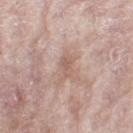Clinical impression:
The lesion was photographed on a routine skin check and not biopsied; there is no pathology result.
Image and clinical context:
On the left thigh. Measured at roughly 3 mm in maximum diameter. A roughly 15 mm field-of-view crop from a total-body skin photograph. Captured under white-light illumination. Automated image analysis of the tile measured an eccentricity of roughly 0.85. The analysis additionally found a lesion–skin lightness drop of about 8 and a normalized lesion–skin contrast near 5.5. And it measured a border-irregularity index near 2.5/10, a color-variation rating of about 1.5/10, and a peripheral color-asymmetry measure near 0.5. The analysis additionally found a classifier nevus-likeness of about 0/100 and lesion-presence confidence of about 95/100. A female subject, aged 68 to 72.